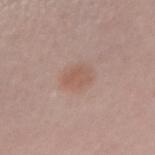notes=catalogued during a skin exam; not biopsied | image source=~15 mm tile from a whole-body skin photo | illumination=white-light | anatomic site=the right forearm | automated metrics=a lesion color around L≈56 a*≈19 b*≈26 in CIELAB, roughly 6 lightness units darker than nearby skin, and a normalized lesion–skin contrast near 5.5 | subject=female, aged 33–37.